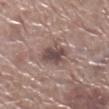Imaged during a routine full-body skin examination; the lesion was not biopsied and no histopathology is available.
This image is a 15 mm lesion crop taken from a total-body photograph.
A male patient roughly 70 years of age.
The lesion is on the right lower leg.